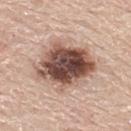Imaged during a routine full-body skin examination; the lesion was not biopsied and no histopathology is available. The lesion is located on the back. The lesion-visualizer software estimated a lesion area of about 29 mm², an outline eccentricity of about 0.6 (0 = round, 1 = elongated), and a symmetry-axis asymmetry near 0.2. The software also gave a mean CIELAB color near L≈50 a*≈19 b*≈25 and a lesion–skin lightness drop of about 21. Captured under white-light illumination. A region of skin cropped from a whole-body photographic capture, roughly 15 mm wide. The patient is a male aged approximately 60.